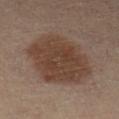Clinical impression: This lesion was catalogued during total-body skin photography and was not selected for biopsy. Context: This is a cross-polarized tile. A female subject aged around 45. Cropped from a whole-body photographic skin survey; the tile spans about 15 mm. An algorithmic analysis of the crop reported an area of roughly 38 mm², an eccentricity of roughly 0.55, and two-axis asymmetry of about 0.15. And it measured a border-irregularity rating of about 2/10 and peripheral color asymmetry of about 1. And it measured a classifier nevus-likeness of about 25/100. The lesion is located on the leg.